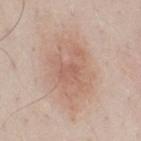Part of a total-body skin-imaging series; this lesion was reviewed on a skin check and was not flagged for biopsy.
A male patient, aged around 50.
On the chest.
The tile uses white-light illumination.
Cropped from a whole-body photographic skin survey; the tile spans about 15 mm.